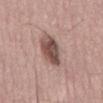workup: catalogued during a skin exam; not biopsied
lighting: white-light
automated lesion analysis: a lesion area of about 16 mm², an eccentricity of roughly 0.85, and a symmetry-axis asymmetry near 0.2; border irregularity of about 3 on a 0–10 scale, a within-lesion color-variation index near 7.5/10, and radial color variation of about 2.5; a nevus-likeness score of about 100/100 and a detector confidence of about 100 out of 100 that the crop contains a lesion
lesion diameter: about 6.5 mm
imaging modality: total-body-photography crop, ~15 mm field of view
subject: male, aged 53 to 57